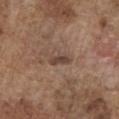Impression: The lesion was tiled from a total-body skin photograph and was not biopsied. Acquisition and patient details: A male subject, aged 73 to 77. About 3 mm across. From the front of the torso. A 15 mm close-up tile from a total-body photography series done for melanoma screening. Automated tile analysis of the lesion measured a footprint of about 3.5 mm², a shape eccentricity near 0.85, and a shape-asymmetry score of about 0.35 (0 = symmetric). The analysis additionally found a lesion color around L≈43 a*≈17 b*≈24 in CIELAB, a lesion–skin lightness drop of about 9, and a normalized border contrast of about 7.5. And it measured a border-irregularity rating of about 3/10, a color-variation rating of about 1.5/10, and a peripheral color-asymmetry measure near 0.5. And it measured a classifier nevus-likeness of about 0/100 and lesion-presence confidence of about 95/100. Imaged with white-light lighting.